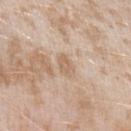| field | value |
|---|---|
| biopsy status | total-body-photography surveillance lesion; no biopsy |
| location | the left upper arm |
| illumination | white-light illumination |
| acquisition | total-body-photography crop, ~15 mm field of view |
| lesion diameter | about 3 mm |
| subject | female, approximately 25 years of age |